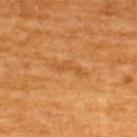Imaged during a routine full-body skin examination; the lesion was not biopsied and no histopathology is available.